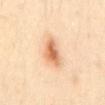follow-up = no biopsy performed (imaged during a skin exam).